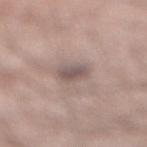Part of a total-body skin-imaging series; this lesion was reviewed on a skin check and was not flagged for biopsy.
A male patient aged 38 to 42.
The tile uses white-light illumination.
On the leg.
The lesion's longest dimension is about 2.5 mm.
Cropped from a whole-body photographic skin survey; the tile spans about 15 mm.